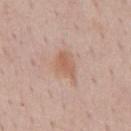{"biopsy_status": "not biopsied; imaged during a skin examination", "patient": {"sex": "male", "age_approx": 50}, "image": {"source": "total-body photography crop", "field_of_view_mm": 15}, "site": "mid back", "lighting": "white-light"}